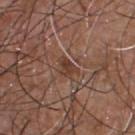Part of a total-body skin-imaging series; this lesion was reviewed on a skin check and was not flagged for biopsy. The lesion is located on the front of the torso. This is a white-light tile. A region of skin cropped from a whole-body photographic capture, roughly 15 mm wide. Measured at roughly 2.5 mm in maximum diameter. The subject is a male approximately 55 years of age. Automated image analysis of the tile measured an automated nevus-likeness rating near 0 out of 100.